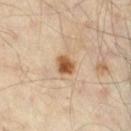{
  "biopsy_status": "not biopsied; imaged during a skin examination",
  "lighting": "cross-polarized",
  "site": "right thigh",
  "patient": {
    "age_approx": 55
  },
  "image": {
    "source": "total-body photography crop",
    "field_of_view_mm": 15
  },
  "automated_metrics": {
    "area_mm2_approx": 4.5,
    "eccentricity": 0.45,
    "cielab_L": 55,
    "cielab_a": 20,
    "cielab_b": 36,
    "vs_skin_darker_L": 15.0,
    "vs_skin_contrast_norm": 10.5,
    "border_irregularity_0_10": 1.5,
    "color_variation_0_10": 4.5,
    "peripheral_color_asymmetry": 1.5,
    "nevus_likeness_0_100": 100,
    "lesion_detection_confidence_0_100": 100
  }
}